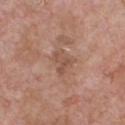A close-up tile cropped from a whole-body skin photograph, about 15 mm across.
Located on the front of the torso.
A male patient approximately 60 years of age.